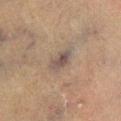follow-up: catalogued during a skin exam; not biopsied | subject: male, about 75 years old | body site: the right lower leg | TBP lesion metrics: a mean CIELAB color near L≈40 a*≈11 b*≈18, a lesion–skin lightness drop of about 7, and a lesion-to-skin contrast of about 7.5 (normalized; higher = more distinct); a border-irregularity rating of about 2.5/10 and a color-variation rating of about 4/10 | tile lighting: cross-polarized | acquisition: 15 mm crop, total-body photography | size: ≈3.5 mm.